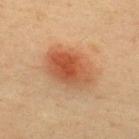This lesion was catalogued during total-body skin photography and was not selected for biopsy. From the upper back. A male patient, about 40 years old. A close-up tile cropped from a whole-body skin photograph, about 15 mm across. An algorithmic analysis of the crop reported a footprint of about 19 mm² and an eccentricity of roughly 0.75. The software also gave a border-irregularity rating of about 2/10 and a peripheral color-asymmetry measure near 2. The recorded lesion diameter is about 6 mm. Imaged with cross-polarized lighting.